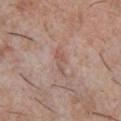biopsy status — imaged on a skin check; not biopsied
site — the chest
imaging modality — ~15 mm tile from a whole-body skin photo
subject — male, aged around 30
lighting — white-light
automated lesion analysis — an area of roughly 3 mm², a shape eccentricity near 0.9, and a shape-asymmetry score of about 0.45 (0 = symmetric); a mean CIELAB color near L≈54 a*≈20 b*≈24, roughly 7 lightness units darker than nearby skin, and a normalized border contrast of about 5.5